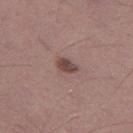Case summary:
* workup · catalogued during a skin exam; not biopsied
* lesion diameter · ~2.5 mm (longest diameter)
* automated metrics · a mean CIELAB color near L≈45 a*≈18 b*≈20, roughly 12 lightness units darker than nearby skin, and a lesion-to-skin contrast of about 9 (normalized; higher = more distinct); a classifier nevus-likeness of about 70/100 and a lesion-detection confidence of about 100/100
* location · the left thigh
* imaging modality · ~15 mm crop, total-body skin-cancer survey
* subject · male, approximately 45 years of age
* lighting · white-light